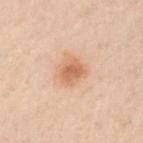  biopsy_status: not biopsied; imaged during a skin examination